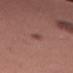| field | value |
|---|---|
| tile lighting | white-light |
| subject | female, roughly 50 years of age |
| image source | ~15 mm tile from a whole-body skin photo |
| anatomic site | the left thigh |
| TBP lesion metrics | about 7 CIELAB-L* units darker than the surrounding skin and a normalized border contrast of about 6; a lesion-detection confidence of about 100/100 |